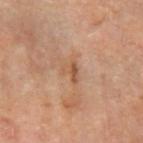Recorded during total-body skin imaging; not selected for excision or biopsy. The patient is a female aged 38–42. From the arm. Longest diameter approximately 2.5 mm. Captured under cross-polarized illumination. A 15 mm close-up extracted from a 3D total-body photography capture.